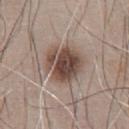No biopsy was performed on this lesion — it was imaged during a full skin examination and was not determined to be concerning. Imaged with white-light lighting. Longest diameter approximately 4.5 mm. The subject is a male aged 63 to 67. A roughly 15 mm field-of-view crop from a total-body skin photograph. The lesion-visualizer software estimated a mean CIELAB color near L≈46 a*≈16 b*≈22, roughly 15 lightness units darker than nearby skin, and a normalized lesion–skin contrast near 11. And it measured border irregularity of about 2.5 on a 0–10 scale and a within-lesion color-variation index near 6/10. From the front of the torso.